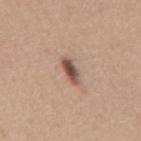follow-up: total-body-photography surveillance lesion; no biopsy.